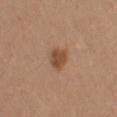The lesion was photographed on a routine skin check and not biopsied; there is no pathology result.
This is a white-light tile.
A region of skin cropped from a whole-body photographic capture, roughly 15 mm wide.
The patient is a female about 40 years old.
The lesion is on the arm.